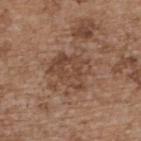Captured during whole-body skin photography for melanoma surveillance; the lesion was not biopsied.